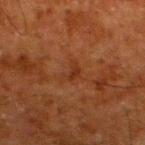follow-up: total-body-photography surveillance lesion; no biopsy
anatomic site: the left lower leg
imaging modality: ~15 mm crop, total-body skin-cancer survey
illumination: cross-polarized
diameter: about 2.5 mm
subject: male, approximately 80 years of age